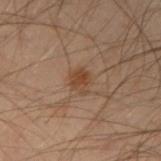Notes:
- workup — total-body-photography surveillance lesion; no biopsy
- TBP lesion metrics — a mean CIELAB color near L≈35 a*≈16 b*≈26 and a normalized lesion–skin contrast near 7.5; a within-lesion color-variation index near 1.5/10 and a peripheral color-asymmetry measure near 0.5
- patient — male, roughly 50 years of age
- diameter — about 2.5 mm
- image — ~15 mm tile from a whole-body skin photo
- body site — the left forearm
- tile lighting — cross-polarized